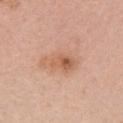<lesion>
  <biopsy_status>not biopsied; imaged during a skin examination</biopsy_status>
  <image>
    <source>total-body photography crop</source>
    <field_of_view_mm>15</field_of_view_mm>
  </image>
  <site>right upper arm</site>
  <lesion_size>
    <long_diameter_mm_approx>5.5</long_diameter_mm_approx>
  </lesion_size>
  <patient>
    <sex>female</sex>
    <age_approx>35</age_approx>
  </patient>
</lesion>